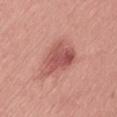Part of a total-body skin-imaging series; this lesion was reviewed on a skin check and was not flagged for biopsy. On the left thigh. A female patient aged 38–42. About 6.5 mm across. An algorithmic analysis of the crop reported an average lesion color of about L≈54 a*≈28 b*≈25 (CIELAB), roughly 12 lightness units darker than nearby skin, and a normalized border contrast of about 7.5. The software also gave an automated nevus-likeness rating near 40 out of 100 and a detector confidence of about 100 out of 100 that the crop contains a lesion. Imaged with white-light lighting. A lesion tile, about 15 mm wide, cut from a 3D total-body photograph.